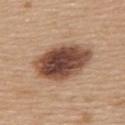Assessment: Recorded during total-body skin imaging; not selected for excision or biopsy. Background: Longest diameter approximately 7 mm. A roughly 15 mm field-of-view crop from a total-body skin photograph. A female subject approximately 65 years of age. Automated image analysis of the tile measured an area of roughly 23 mm², an eccentricity of roughly 0.8, and two-axis asymmetry of about 0.1. It also reported a lesion color around L≈46 a*≈20 b*≈28 in CIELAB, a lesion–skin lightness drop of about 19, and a lesion-to-skin contrast of about 13.5 (normalized; higher = more distinct). It also reported a border-irregularity rating of about 1.5/10, a color-variation rating of about 7.5/10, and a peripheral color-asymmetry measure near 2. On the upper back. Imaged with white-light lighting.